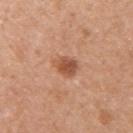Q: Is there a histopathology result?
A: total-body-photography surveillance lesion; no biopsy
Q: Where on the body is the lesion?
A: the right upper arm
Q: What is the imaging modality?
A: 15 mm crop, total-body photography
Q: What are the patient's age and sex?
A: female, in their 50s
Q: How large is the lesion?
A: ~3 mm (longest diameter)
Q: What lighting was used for the tile?
A: white-light illumination